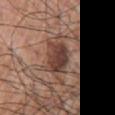Notes:
• notes: no biopsy performed (imaged during a skin exam)
• location: the left arm
• acquisition: total-body-photography crop, ~15 mm field of view
• lesion diameter: ~4 mm (longest diameter)
• tile lighting: white-light
• subject: male, approximately 70 years of age